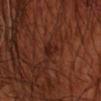Assessment:
No biopsy was performed on this lesion — it was imaged during a full skin examination and was not determined to be concerning.
Acquisition and patient details:
The lesion is located on the left forearm. Measured at roughly 2.5 mm in maximum diameter. A male subject, about 70 years old. Automated image analysis of the tile measured a border-irregularity rating of about 3/10, internal color variation of about 1.5 on a 0–10 scale, and a peripheral color-asymmetry measure near 0.5. It also reported a classifier nevus-likeness of about 0/100 and a lesion-detection confidence of about 95/100. Cropped from a total-body skin-imaging series; the visible field is about 15 mm.